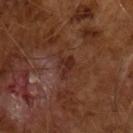Clinical impression: The lesion was tiled from a total-body skin photograph and was not biopsied. Acquisition and patient details: The subject is a male aged approximately 65. A 15 mm close-up extracted from a 3D total-body photography capture. The tile uses cross-polarized illumination.